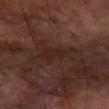Q: Patient demographics?
A: male, roughly 60 years of age
Q: How large is the lesion?
A: ~4 mm (longest diameter)
Q: What is the imaging modality?
A: 15 mm crop, total-body photography
Q: How was the tile lit?
A: cross-polarized
Q: What is the anatomic site?
A: the right forearm